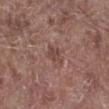<record>
<biopsy_status>not biopsied; imaged during a skin examination</biopsy_status>
<site>right lower leg</site>
<patient>
  <sex>male</sex>
  <age_approx>55</age_approx>
</patient>
<image>
  <source>total-body photography crop</source>
  <field_of_view_mm>15</field_of_view_mm>
</image>
</record>